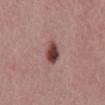Clinical summary: A 15 mm close-up tile from a total-body photography series done for melanoma screening. The lesion's longest dimension is about 3.5 mm. A male subject approximately 40 years of age. The tile uses white-light illumination. Located on the mid back.